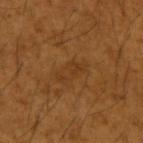Assessment: Captured during whole-body skin photography for melanoma surveillance; the lesion was not biopsied. Context: The recorded lesion diameter is about 3.5 mm. A male subject aged 63 to 67. From the left upper arm. A 15 mm close-up extracted from a 3D total-body photography capture.